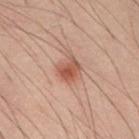{"biopsy_status": "not biopsied; imaged during a skin examination", "patient": {"sex": "male", "age_approx": 40}, "image": {"source": "total-body photography crop", "field_of_view_mm": 15}, "site": "abdomen", "lighting": "cross-polarized"}